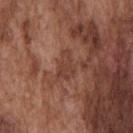Impression:
Part of a total-body skin-imaging series; this lesion was reviewed on a skin check and was not flagged for biopsy.
Context:
The tile uses white-light illumination. A lesion tile, about 15 mm wide, cut from a 3D total-body photograph. About 3.5 mm across. The subject is a male about 75 years old. The lesion is on the chest. An algorithmic analysis of the crop reported a lesion color around L≈41 a*≈22 b*≈27 in CIELAB. And it measured a classifier nevus-likeness of about 0/100 and a detector confidence of about 70 out of 100 that the crop contains a lesion.